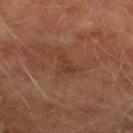Impression:
The lesion was tiled from a total-body skin photograph and was not biopsied.
Context:
A 15 mm close-up extracted from a 3D total-body photography capture. A male subject, aged approximately 75. On the leg.